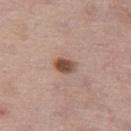Impression:
This lesion was catalogued during total-body skin photography and was not selected for biopsy.